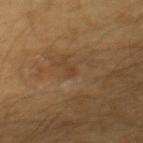No biopsy was performed on this lesion — it was imaged during a full skin examination and was not determined to be concerning. Approximately 1.5 mm at its widest. From the upper back. A 15 mm close-up tile from a total-body photography series done for melanoma screening. Automated image analysis of the tile measured a shape eccentricity near 0.75 and a symmetry-axis asymmetry near 0.3. The analysis additionally found a lesion–skin lightness drop of about 5 and a normalized lesion–skin contrast near 5. A male subject, aged approximately 60. The tile uses cross-polarized illumination.